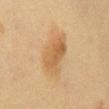site = the front of the torso | tile lighting = cross-polarized | acquisition = ~15 mm tile from a whole-body skin photo | subject = female, aged approximately 50.